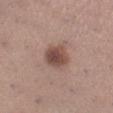Part of a total-body skin-imaging series; this lesion was reviewed on a skin check and was not flagged for biopsy.